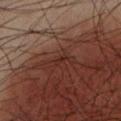biopsy_status: not biopsied; imaged during a skin examination
image:
  source: total-body photography crop
  field_of_view_mm: 15
patient:
  sex: male
  age_approx: 40
lesion_size:
  long_diameter_mm_approx: 1.0
site: left lower leg